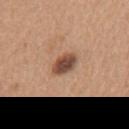Clinical impression:
The lesion was photographed on a routine skin check and not biopsied; there is no pathology result.
Background:
The tile uses white-light illumination. The recorded lesion diameter is about 3.5 mm. Automated image analysis of the tile measured a lesion area of about 6 mm², an outline eccentricity of about 0.8 (0 = round, 1 = elongated), and two-axis asymmetry of about 0.2. It also reported a lesion color around L≈48 a*≈20 b*≈28 in CIELAB and a normalized border contrast of about 11. The software also gave a border-irregularity index near 2/10, a color-variation rating of about 4.5/10, and radial color variation of about 1. A female subject, aged around 50. The lesion is on the mid back. A 15 mm close-up extracted from a 3D total-body photography capture.